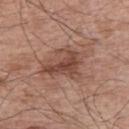workup: no biopsy performed (imaged during a skin exam); lighting: white-light illumination; location: the right upper arm; subject: male, aged approximately 65; lesion size: ≈5 mm; image source: 15 mm crop, total-body photography.